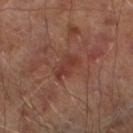Notes:
* biopsy status · catalogued during a skin exam; not biopsied
* location · the left lower leg
* patient · male, about 65 years old
* lesion diameter · ≈3 mm
* lighting · cross-polarized illumination
* image source · total-body-photography crop, ~15 mm field of view